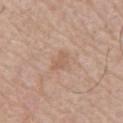| feature | finding |
|---|---|
| workup | total-body-photography surveillance lesion; no biopsy |
| anatomic site | the chest |
| tile lighting | white-light |
| automated metrics | a footprint of about 3.5 mm², an outline eccentricity of about 0.8 (0 = round, 1 = elongated), and a shape-asymmetry score of about 0.25 (0 = symmetric); a lesion–skin lightness drop of about 6 and a lesion-to-skin contrast of about 5 (normalized; higher = more distinct); a border-irregularity index near 2.5/10 and a within-lesion color-variation index near 1.5/10; an automated nevus-likeness rating near 0 out of 100 |
| patient | male, in their mid- to late 70s |
| lesion diameter | ≈2.5 mm |
| image source | ~15 mm tile from a whole-body skin photo |